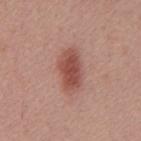Impression:
Part of a total-body skin-imaging series; this lesion was reviewed on a skin check and was not flagged for biopsy.
Context:
From the back. A roughly 15 mm field-of-view crop from a total-body skin photograph. The subject is a male approximately 55 years of age.